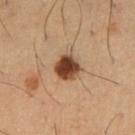Findings:
– follow-up · total-body-photography surveillance lesion; no biopsy
– imaging modality · ~15 mm tile from a whole-body skin photo
– subject · male, approximately 40 years of age
– body site · the right upper arm